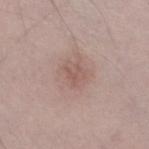Assessment:
The lesion was photographed on a routine skin check and not biopsied; there is no pathology result.
Context:
The lesion is located on the leg. Cropped from a whole-body photographic skin survey; the tile spans about 15 mm. About 2 mm across. Imaged with white-light lighting. Automated tile analysis of the lesion measured a lesion color around L≈55 a*≈19 b*≈23 in CIELAB and roughly 7 lightness units darker than nearby skin. And it measured a border-irregularity index near 5.5/10, a color-variation rating of about 0/10, and radial color variation of about 0. The subject is a male approximately 45 years of age.